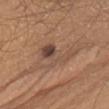Clinical impression: The lesion was tiled from a total-body skin photograph and was not biopsied. Background: The recorded lesion diameter is about 5.5 mm. The lesion is located on the chest. A close-up tile cropped from a whole-body skin photograph, about 15 mm across. This is a white-light tile. A male patient aged 33 to 37.